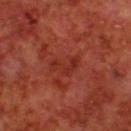biopsy status: catalogued during a skin exam; not biopsied | body site: the upper back | acquisition: 15 mm crop, total-body photography | subject: male, about 70 years old.